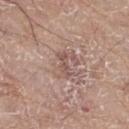Case summary:
- biopsy status — imaged on a skin check; not biopsied
- automated metrics — a border-irregularity index near 5/10 and peripheral color asymmetry of about 0
- anatomic site — the left leg
- image source — 15 mm crop, total-body photography
- subject — male, aged around 80